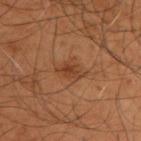Impression:
The lesion was tiled from a total-body skin photograph and was not biopsied.
Context:
The lesion-visualizer software estimated an area of roughly 5.5 mm², an outline eccentricity of about 0.7 (0 = round, 1 = elongated), and a symmetry-axis asymmetry near 0.35. The analysis additionally found a lesion color around L≈40 a*≈22 b*≈33 in CIELAB, a lesion–skin lightness drop of about 7, and a lesion-to-skin contrast of about 6 (normalized; higher = more distinct). On the upper back. Measured at roughly 3.5 mm in maximum diameter. A roughly 15 mm field-of-view crop from a total-body skin photograph. A male subject in their mid-50s. Imaged with cross-polarized lighting.